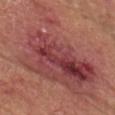<tbp_lesion>
<biopsy_status>not biopsied; imaged during a skin examination</biopsy_status>
<automated_metrics>
  <cielab_L>45</cielab_L>
  <cielab_a>28</cielab_a>
  <cielab_b>23</cielab_b>
  <vs_skin_darker_L>9.0</vs_skin_darker_L>
  <vs_skin_contrast_norm>8.0</vs_skin_contrast_norm>
  <border_irregularity_0_10>6.5</border_irregularity_0_10>
  <color_variation_0_10>9.5</color_variation_0_10>
  <peripheral_color_asymmetry>3.5</peripheral_color_asymmetry>
  <nevus_likeness_0_100>0</nevus_likeness_0_100>
  <lesion_detection_confidence_0_100>95</lesion_detection_confidence_0_100>
</automated_metrics>
<lesion_size>
  <long_diameter_mm_approx>16.0</long_diameter_mm_approx>
</lesion_size>
<lighting>white-light</lighting>
<image>
  <source>total-body photography crop</source>
  <field_of_view_mm>15</field_of_view_mm>
</image>
<patient>
  <sex>male</sex>
  <age_approx>65</age_approx>
</patient>
<site>chest</site>
</tbp_lesion>